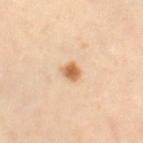No biopsy was performed on this lesion — it was imaged during a full skin examination and was not determined to be concerning. The total-body-photography lesion software estimated about 14 CIELAB-L* units darker than the surrounding skin and a normalized lesion–skin contrast near 9. The analysis additionally found radial color variation of about 1.5. A close-up tile cropped from a whole-body skin photograph, about 15 mm across. A female patient, approximately 40 years of age. The lesion is on the right thigh.